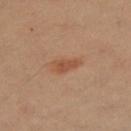Recorded during total-body skin imaging; not selected for excision or biopsy. From the right thigh. The subject is a female approximately 50 years of age. The total-body-photography lesion software estimated a lesion area of about 4.5 mm². The analysis additionally found a lesion color around L≈49 a*≈22 b*≈31 in CIELAB and a normalized border contrast of about 6.5. It also reported an automated nevus-likeness rating near 60 out of 100 and a lesion-detection confidence of about 100/100. A lesion tile, about 15 mm wide, cut from a 3D total-body photograph.